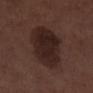Located on the left lower leg. Measured at roughly 7 mm in maximum diameter. A lesion tile, about 15 mm wide, cut from a 3D total-body photograph. A male subject, in their 70s.